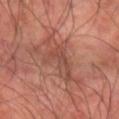Q: Lesion location?
A: the left forearm
Q: What did automated image analysis measure?
A: a lesion area of about 13 mm² and a shape-asymmetry score of about 0.5 (0 = symmetric); an average lesion color of about L≈48 a*≈24 b*≈28 (CIELAB), about 7 CIELAB-L* units darker than the surrounding skin, and a normalized border contrast of about 5.5; a border-irregularity index near 8.5/10 and radial color variation of about 1.5
Q: What are the patient's age and sex?
A: male, aged 63–67
Q: What is the imaging modality?
A: total-body-photography crop, ~15 mm field of view
Q: How large is the lesion?
A: ~6.5 mm (longest diameter)
Q: Illumination type?
A: cross-polarized illumination